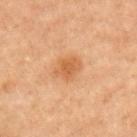<record>
  <biopsy_status>not biopsied; imaged during a skin examination</biopsy_status>
  <lesion_size>
    <long_diameter_mm_approx>3.0</long_diameter_mm_approx>
  </lesion_size>
  <image>
    <source>total-body photography crop</source>
    <field_of_view_mm>15</field_of_view_mm>
  </image>
  <lighting>cross-polarized</lighting>
  <site>right upper arm</site>
  <patient>
    <sex>male</sex>
    <age_approx>70</age_approx>
  </patient>
</record>